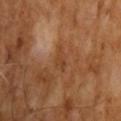Clinical impression:
Part of a total-body skin-imaging series; this lesion was reviewed on a skin check and was not flagged for biopsy.
Image and clinical context:
A 15 mm close-up extracted from a 3D total-body photography capture. The recorded lesion diameter is about 3.5 mm. Imaged with cross-polarized lighting. A male patient in their mid- to late 60s.